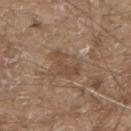Notes:
* follow-up · catalogued during a skin exam; not biopsied
* patient · male, aged around 80
* image · total-body-photography crop, ~15 mm field of view
* body site · the upper back